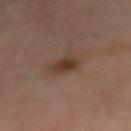No biopsy was performed on this lesion — it was imaged during a full skin examination and was not determined to be concerning. Located on the mid back. This is a cross-polarized tile. Cropped from a total-body skin-imaging series; the visible field is about 15 mm. A male subject aged around 50. Longest diameter approximately 2.5 mm.